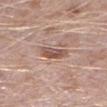A male subject aged approximately 50.
The lesion is located on the leg.
The lesion-visualizer software estimated a footprint of about 5 mm², an outline eccentricity of about 0.85 (0 = round, 1 = elongated), and a symmetry-axis asymmetry near 0.25. And it measured a mean CIELAB color near L≈53 a*≈20 b*≈26, a lesion–skin lightness drop of about 12, and a normalized lesion–skin contrast near 8. It also reported a peripheral color-asymmetry measure near 1.
A region of skin cropped from a whole-body photographic capture, roughly 15 mm wide.